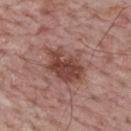Notes:
• follow-up: total-body-photography surveillance lesion; no biopsy
• tile lighting: white-light
• location: the upper back
• image: ~15 mm crop, total-body skin-cancer survey
• size: ~5.5 mm (longest diameter)
• patient: male, aged around 65